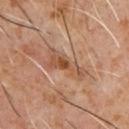Part of a total-body skin-imaging series; this lesion was reviewed on a skin check and was not flagged for biopsy. This image is a 15 mm lesion crop taken from a total-body photograph. The subject is a male approximately 60 years of age. The lesion is on the chest.